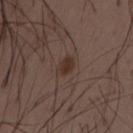On the left thigh. A male patient, aged around 50. A lesion tile, about 15 mm wide, cut from a 3D total-body photograph. Longest diameter approximately 2.5 mm. Captured under white-light illumination.